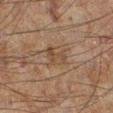Captured during whole-body skin photography for melanoma surveillance; the lesion was not biopsied. A close-up tile cropped from a whole-body skin photograph, about 15 mm across. Located on the left leg. A male patient about 60 years old.